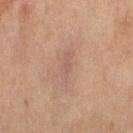Q: Was this lesion biopsied?
A: no biopsy performed (imaged during a skin exam)
Q: What lighting was used for the tile?
A: cross-polarized
Q: What is the anatomic site?
A: the right thigh
Q: Automated lesion metrics?
A: a footprint of about 4.5 mm² and two-axis asymmetry of about 0.3; a mean CIELAB color near L≈50 a*≈17 b*≈23, about 5 CIELAB-L* units darker than the surrounding skin, and a lesion-to-skin contrast of about 4.5 (normalized; higher = more distinct); a nevus-likeness score of about 0/100 and lesion-presence confidence of about 75/100
Q: Patient demographics?
A: female, about 60 years old
Q: What kind of image is this?
A: ~15 mm tile from a whole-body skin photo
Q: What is the lesion's diameter?
A: about 4 mm